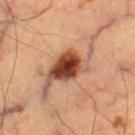<tbp_lesion>
  <biopsy_status>not biopsied; imaged during a skin examination</biopsy_status>
  <automated_metrics>
    <cielab_L>39</cielab_L>
    <cielab_a>19</cielab_a>
    <cielab_b>26</cielab_b>
    <vs_skin_darker_L>13.0</vs_skin_darker_L>
    <vs_skin_contrast_norm>11.0</vs_skin_contrast_norm>
    <color_variation_0_10>8.5</color_variation_0_10>
    <peripheral_color_asymmetry>2.0</peripheral_color_asymmetry>
    <nevus_likeness_0_100>100</nevus_likeness_0_100>
  </automated_metrics>
  <lighting>cross-polarized</lighting>
  <patient>
    <sex>male</sex>
    <age_approx>65</age_approx>
  </patient>
  <lesion_size>
    <long_diameter_mm_approx>8.0</long_diameter_mm_approx>
  </lesion_size>
  <image>
    <source>total-body photography crop</source>
    <field_of_view_mm>15</field_of_view_mm>
  </image>
  <site>right thigh</site>
</tbp_lesion>